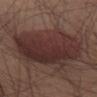| field | value |
|---|---|
| notes | no biopsy performed (imaged during a skin exam) |
| acquisition | 15 mm crop, total-body photography |
| site | the abdomen |
| patient | male, in their 50s |